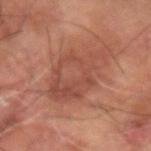Q: Is there a histopathology result?
A: total-body-photography surveillance lesion; no biopsy
Q: How was this image acquired?
A: total-body-photography crop, ~15 mm field of view
Q: What are the patient's age and sex?
A: male, about 60 years old
Q: What did automated image analysis measure?
A: an automated nevus-likeness rating near 0 out of 100
Q: What is the anatomic site?
A: the right arm